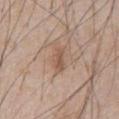No biopsy was performed on this lesion — it was imaged during a full skin examination and was not determined to be concerning.
On the abdomen.
This is a white-light tile.
A close-up tile cropped from a whole-body skin photograph, about 15 mm across.
The patient is a male about 65 years old.
The recorded lesion diameter is about 3 mm.